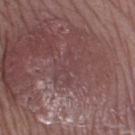Assessment: The lesion was tiled from a total-body skin photograph and was not biopsied. Context: From the leg. A 15 mm crop from a total-body photograph taken for skin-cancer surveillance. The lesion-visualizer software estimated an outline eccentricity of about 0.65 (0 = round, 1 = elongated) and two-axis asymmetry of about 0.6. This is a white-light tile. The subject is a male in their 70s. Approximately 3.5 mm at its widest.